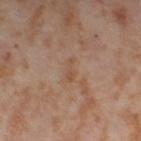<tbp_lesion>
  <biopsy_status>not biopsied; imaged during a skin examination</biopsy_status>
  <site>leg</site>
  <lesion_size>
    <long_diameter_mm_approx>2.5</long_diameter_mm_approx>
  </lesion_size>
  <image>
    <source>total-body photography crop</source>
    <field_of_view_mm>15</field_of_view_mm>
  </image>
  <patient>
    <sex>female</sex>
    <age_approx>55</age_approx>
  </patient>
  <lighting>cross-polarized</lighting>
</tbp_lesion>